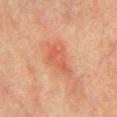follow-up: total-body-photography surveillance lesion; no biopsy | tile lighting: cross-polarized illumination | patient: male, aged around 75 | location: the chest | acquisition: 15 mm crop, total-body photography.